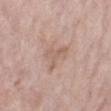Clinical impression:
Part of a total-body skin-imaging series; this lesion was reviewed on a skin check and was not flagged for biopsy.
Image and clinical context:
The lesion-visualizer software estimated an average lesion color of about L≈60 a*≈18 b*≈26 (CIELAB) and a normalized lesion–skin contrast near 5. The analysis additionally found a border-irregularity index near 7/10, a color-variation rating of about 2/10, and a peripheral color-asymmetry measure near 0.5. A female patient, about 70 years old. The tile uses white-light illumination. Measured at roughly 3.5 mm in maximum diameter. A roughly 15 mm field-of-view crop from a total-body skin photograph. The lesion is located on the left lower leg.